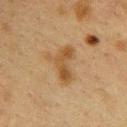Part of a total-body skin-imaging series; this lesion was reviewed on a skin check and was not flagged for biopsy.
The lesion is on the upper back.
Imaged with cross-polarized lighting.
The recorded lesion diameter is about 4.5 mm.
The patient is a female aged around 40.
A 15 mm close-up tile from a total-body photography series done for melanoma screening.
The lesion-visualizer software estimated a border-irregularity rating of about 6/10, internal color variation of about 3.5 on a 0–10 scale, and peripheral color asymmetry of about 1. And it measured a classifier nevus-likeness of about 0/100.